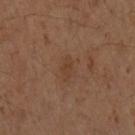Imaged during a routine full-body skin examination; the lesion was not biopsied and no histopathology is available. Measured at roughly 2.5 mm in maximum diameter. A close-up tile cropped from a whole-body skin photograph, about 15 mm across. Located on the left forearm. Automated tile analysis of the lesion measured an area of roughly 3 mm², an eccentricity of roughly 0.8, and two-axis asymmetry of about 0.4. It also reported an average lesion color of about L≈31 a*≈16 b*≈24 (CIELAB), a lesion–skin lightness drop of about 4, and a normalized border contrast of about 4.5. It also reported border irregularity of about 3.5 on a 0–10 scale, a within-lesion color-variation index near 2/10, and peripheral color asymmetry of about 1. Captured under cross-polarized illumination. The patient is a female roughly 55 years of age.